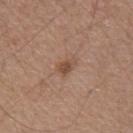{"biopsy_status": "not biopsied; imaged during a skin examination", "automated_metrics": {"cielab_L": 49, "cielab_a": 19, "cielab_b": 29, "vs_skin_darker_L": 9.0, "vs_skin_contrast_norm": 7.0, "border_irregularity_0_10": 3.0, "color_variation_0_10": 2.5, "peripheral_color_asymmetry": 1.0}, "patient": {"sex": "male", "age_approx": 55}, "lighting": "white-light", "site": "left upper arm", "image": {"source": "total-body photography crop", "field_of_view_mm": 15}}